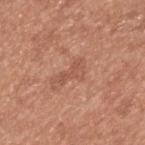Clinical impression: The lesion was tiled from a total-body skin photograph and was not biopsied. Background: Located on the upper back. A male patient approximately 55 years of age. Automated image analysis of the tile measured a detector confidence of about 100 out of 100 that the crop contains a lesion. Cropped from a total-body skin-imaging series; the visible field is about 15 mm. About 3.5 mm across. This is a white-light tile.